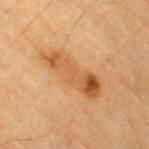| field | value |
|---|---|
| notes | catalogued during a skin exam; not biopsied |